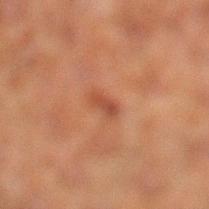Clinical impression: Imaged during a routine full-body skin examination; the lesion was not biopsied and no histopathology is available. Image and clinical context: The lesion is located on the leg. Approximately 2.5 mm at its widest. This is a cross-polarized tile. A male subject, aged 48 to 52. A lesion tile, about 15 mm wide, cut from a 3D total-body photograph.